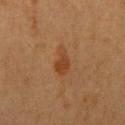A close-up tile cropped from a whole-body skin photograph, about 15 mm across. The lesion's longest dimension is about 3 mm. A female patient, in their 50s. This is a cross-polarized tile. From the right upper arm.